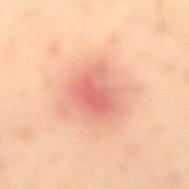workup = imaged on a skin check; not biopsied
diameter = about 4.5 mm
automated lesion analysis = a lesion area of about 15 mm², a shape eccentricity near 0.45, and two-axis asymmetry of about 0.2; a lesion–skin lightness drop of about 10; border irregularity of about 2.5 on a 0–10 scale, internal color variation of about 6 on a 0–10 scale, and radial color variation of about 2; lesion-presence confidence of about 100/100
anatomic site = the lower back
subject = male, aged approximately 55
image source = ~15 mm crop, total-body skin-cancer survey
lighting = cross-polarized